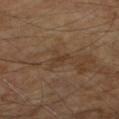Notes:
• site · the arm
• subject · male, aged around 70
• image source · total-body-photography crop, ~15 mm field of view